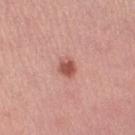location: the right lower leg | illumination: white-light | patient: female, aged approximately 25 | image: ~15 mm crop, total-body skin-cancer survey | size: ≈2.5 mm.